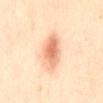biopsy status = total-body-photography surveillance lesion; no biopsy | image source = ~15 mm tile from a whole-body skin photo | subject = female, about 40 years old | tile lighting = cross-polarized | location = the abdomen | automated metrics = an average lesion color of about L≈73 a*≈24 b*≈37 (CIELAB) and a normalized border contrast of about 7.5; a lesion-detection confidence of about 100/100.